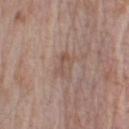This lesion was catalogued during total-body skin photography and was not selected for biopsy.
A lesion tile, about 15 mm wide, cut from a 3D total-body photograph.
A male patient aged around 70.
Captured under white-light illumination.
Measured at roughly 3 mm in maximum diameter.
The lesion is on the arm.
The total-body-photography lesion software estimated a lesion color around L≈52 a*≈17 b*≈25 in CIELAB, roughly 7 lightness units darker than nearby skin, and a normalized border contrast of about 5.